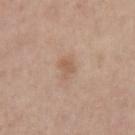Q: Was this lesion biopsied?
A: catalogued during a skin exam; not biopsied
Q: What is the imaging modality?
A: ~15 mm crop, total-body skin-cancer survey
Q: Illumination type?
A: white-light
Q: Lesion location?
A: the arm
Q: Patient demographics?
A: female, aged 38–42
Q: Lesion size?
A: about 2.5 mm